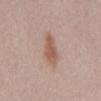{"biopsy_status": "not biopsied; imaged during a skin examination", "automated_metrics": {"area_mm2_approx": 7.5, "eccentricity": 0.9, "cielab_L": 57, "cielab_a": 20, "cielab_b": 27, "vs_skin_darker_L": 10.0}, "site": "mid back", "image": {"source": "total-body photography crop", "field_of_view_mm": 15}, "lesion_size": {"long_diameter_mm_approx": 4.5}, "lighting": "white-light", "patient": {"sex": "female", "age_approx": 50}}